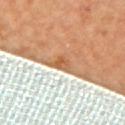Assessment: Imaged during a routine full-body skin examination; the lesion was not biopsied and no histopathology is available. Context: The subject is female. The lesion is located on the upper back. Approximately 2.5 mm at its widest. This image is a 15 mm lesion crop taken from a total-body photograph.